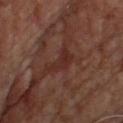{
  "biopsy_status": "not biopsied; imaged during a skin examination",
  "site": "front of the torso",
  "image": {
    "source": "total-body photography crop",
    "field_of_view_mm": 15
  },
  "patient": {
    "sex": "male",
    "age_approx": 65
  }
}